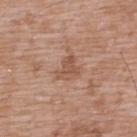This lesion was catalogued during total-body skin photography and was not selected for biopsy. The subject is a male about 50 years old. Longest diameter approximately 3 mm. Cropped from a total-body skin-imaging series; the visible field is about 15 mm. The lesion is located on the upper back. Imaged with white-light lighting.